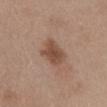Q: Was a biopsy performed?
A: catalogued during a skin exam; not biopsied
Q: What is the lesion's diameter?
A: about 4 mm
Q: What is the imaging modality?
A: ~15 mm crop, total-body skin-cancer survey
Q: Who is the patient?
A: female, aged around 55
Q: What is the anatomic site?
A: the front of the torso